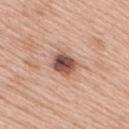Clinical summary: A male patient aged approximately 60. Located on the right upper arm. Cropped from a whole-body photographic skin survey; the tile spans about 15 mm.